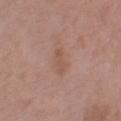notes = catalogued during a skin exam; not biopsied | lighting = white-light | anatomic site = the right thigh | patient = male, roughly 75 years of age | size = ≈3 mm | acquisition = ~15 mm tile from a whole-body skin photo.